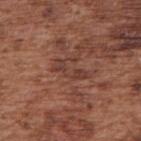Clinical summary: The lesion-visualizer software estimated border irregularity of about 7 on a 0–10 scale, a color-variation rating of about 1.5/10, and radial color variation of about 0.5. The patient is a male about 75 years old. A 15 mm close-up extracted from a 3D total-body photography capture. The lesion is located on the right upper arm. Approximately 4.5 mm at its widest. This is a white-light tile.